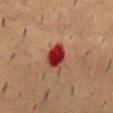{
  "biopsy_status": "not biopsied; imaged during a skin examination",
  "image": {
    "source": "total-body photography crop",
    "field_of_view_mm": 15
  },
  "automated_metrics": {
    "cielab_L": 32,
    "cielab_a": 34,
    "cielab_b": 26,
    "vs_skin_darker_L": 16.0,
    "vs_skin_contrast_norm": 14.0,
    "nevus_likeness_0_100": 0,
    "lesion_detection_confidence_0_100": 100
  },
  "patient": {
    "sex": "male",
    "age_approx": 55
  },
  "lighting": "cross-polarized",
  "site": "mid back",
  "lesion_size": {
    "long_diameter_mm_approx": 3.5
  }
}